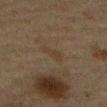Findings:
- notes: no biopsy performed (imaged during a skin exam)
- imaging modality: total-body-photography crop, ~15 mm field of view
- automated metrics: a lesion area of about 4 mm², an eccentricity of roughly 0.9, and two-axis asymmetry of about 0.4; a border-irregularity rating of about 4.5/10, a within-lesion color-variation index near 1/10, and a peripheral color-asymmetry measure near 0.5; a classifier nevus-likeness of about 0/100 and a detector confidence of about 95 out of 100 that the crop contains a lesion
- size: ~3.5 mm (longest diameter)
- location: the abdomen
- patient: male, about 75 years old
- lighting: cross-polarized illumination